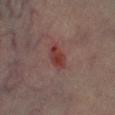biopsy status = total-body-photography surveillance lesion; no biopsy